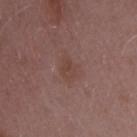notes = no biopsy performed (imaged during a skin exam)
site = the arm
image-analysis metrics = a lesion color around L≈42 a*≈19 b*≈23 in CIELAB, about 5 CIELAB-L* units darker than the surrounding skin, and a normalized lesion–skin contrast near 5.5; a nevus-likeness score of about 0/100 and lesion-presence confidence of about 100/100
image source = ~15 mm tile from a whole-body skin photo
patient = female, roughly 45 years of age